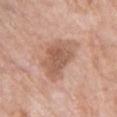A female patient aged 58 to 62. From the front of the torso. A roughly 15 mm field-of-view crop from a total-body skin photograph.